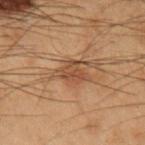Case summary:
• follow-up · catalogued during a skin exam; not biopsied
• subject · male, aged around 55
• image source · 15 mm crop, total-body photography
• anatomic site · the left forearm